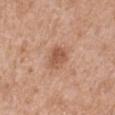Impression: The lesion was tiled from a total-body skin photograph and was not biopsied. Acquisition and patient details: About 3 mm across. From the right upper arm. A male patient aged approximately 65. A lesion tile, about 15 mm wide, cut from a 3D total-body photograph.